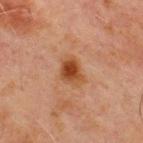Case summary:
* workup · catalogued during a skin exam; not biopsied
* subject · male, aged 68 to 72
* site · the chest
* imaging modality · ~15 mm tile from a whole-body skin photo
* tile lighting · cross-polarized